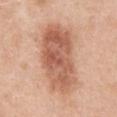Case summary:
– TBP lesion metrics: a footprint of about 35 mm², a shape eccentricity near 0.85, and two-axis asymmetry of about 0.2
– image source: ~15 mm tile from a whole-body skin photo
– site: the abdomen
– subject: female, approximately 40 years of age
– diameter: ≈9 mm
– tile lighting: white-light